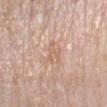| feature | finding |
|---|---|
| biopsy status | total-body-photography surveillance lesion; no biopsy |
| image source | ~15 mm tile from a whole-body skin photo |
| anatomic site | the left forearm |
| patient | female, in their 60s |